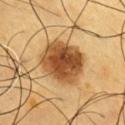notes = no biopsy performed (imaged during a skin exam); anatomic site = the front of the torso; diameter = ≈5.5 mm; subject = male, in their 60s; lighting = cross-polarized; imaging modality = ~15 mm crop, total-body skin-cancer survey.